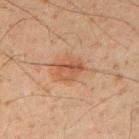Assessment: The lesion was photographed on a routine skin check and not biopsied; there is no pathology result. Image and clinical context: The lesion is on the right upper arm. A 15 mm crop from a total-body photograph taken for skin-cancer surveillance. A male subject, approximately 50 years of age.